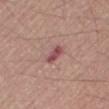Findings:
• workup: total-body-photography surveillance lesion; no biopsy
• anatomic site: the right thigh
• image: total-body-photography crop, ~15 mm field of view
• subject: male, in their mid-60s